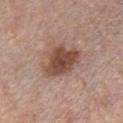Findings:
– biopsy status — total-body-photography surveillance lesion; no biopsy
– site — the chest
– imaging modality — 15 mm crop, total-body photography
– size — ~5.5 mm (longest diameter)
– patient — male, approximately 60 years of age
– automated metrics — a footprint of about 14 mm², a shape eccentricity near 0.7, and a shape-asymmetry score of about 0.3 (0 = symmetric)
– illumination — white-light